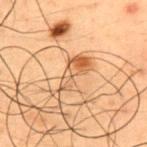Clinical impression:
Imaged during a routine full-body skin examination; the lesion was not biopsied and no histopathology is available.
Acquisition and patient details:
A 15 mm close-up tile from a total-body photography series done for melanoma screening. The lesion is on the back. A male patient, approximately 50 years of age.